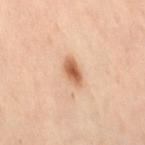Part of a total-body skin-imaging series; this lesion was reviewed on a skin check and was not flagged for biopsy. About 3.5 mm across. Automated tile analysis of the lesion measured an eccentricity of roughly 0.85 and a symmetry-axis asymmetry near 0.25. And it measured a mean CIELAB color near L≈64 a*≈23 b*≈36 and a lesion-to-skin contrast of about 9 (normalized; higher = more distinct). And it measured internal color variation of about 4 on a 0–10 scale and radial color variation of about 1. And it measured an automated nevus-likeness rating near 100 out of 100 and a detector confidence of about 100 out of 100 that the crop contains a lesion. This is a cross-polarized tile. Cropped from a whole-body photographic skin survey; the tile spans about 15 mm. From the left leg. A female subject, aged 38 to 42.